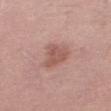Findings:
– biopsy status — catalogued during a skin exam; not biopsied
– automated metrics — a lesion area of about 8 mm², an eccentricity of roughly 0.65, and two-axis asymmetry of about 0.4
– location — the right thigh
– subject — female, about 65 years old
– acquisition — total-body-photography crop, ~15 mm field of view